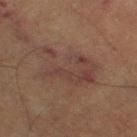Q: Was a biopsy performed?
A: catalogued during a skin exam; not biopsied
Q: How was this image acquired?
A: total-body-photography crop, ~15 mm field of view
Q: Automated lesion metrics?
A: a mean CIELAB color near L≈31 a*≈15 b*≈19, a lesion–skin lightness drop of about 5, and a lesion-to-skin contrast of about 6 (normalized; higher = more distinct)
Q: How was the tile lit?
A: cross-polarized illumination
Q: What are the patient's age and sex?
A: female, aged approximately 60
Q: Lesion size?
A: ~7 mm (longest diameter)
Q: Lesion location?
A: the leg